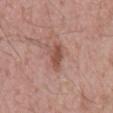Captured during whole-body skin photography for melanoma surveillance; the lesion was not biopsied. A region of skin cropped from a whole-body photographic capture, roughly 15 mm wide. The lesion is located on the back. The recorded lesion diameter is about 3.5 mm. A male subject in their 60s. Imaged with white-light lighting.